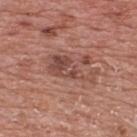biopsy status: catalogued during a skin exam; not biopsied
location: the upper back
patient: male, aged around 70
imaging modality: 15 mm crop, total-body photography